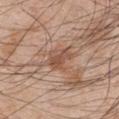The lesion was photographed on a routine skin check and not biopsied; there is no pathology result.
Captured under white-light illumination.
A roughly 15 mm field-of-view crop from a total-body skin photograph.
A male patient, aged 48–52.
The total-body-photography lesion software estimated an automated nevus-likeness rating near 0 out of 100 and a detector confidence of about 100 out of 100 that the crop contains a lesion.
Approximately 3 mm at its widest.
From the left upper arm.